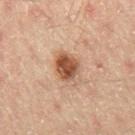Clinical impression: This lesion was catalogued during total-body skin photography and was not selected for biopsy. Acquisition and patient details: A male subject, about 45 years old. A 15 mm close-up extracted from a 3D total-body photography capture. About 3.5 mm across. Located on the leg. This is a cross-polarized tile.